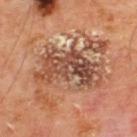Q: Was a biopsy performed?
A: catalogued during a skin exam; not biopsied
Q: Lesion location?
A: the upper back
Q: What lighting was used for the tile?
A: cross-polarized illumination
Q: What is the lesion's diameter?
A: ~8 mm (longest diameter)
Q: Patient demographics?
A: male, in their mid-60s
Q: What kind of image is this?
A: ~15 mm crop, total-body skin-cancer survey